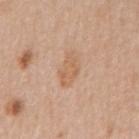follow-up — catalogued during a skin exam; not biopsied | illumination — white-light | acquisition — total-body-photography crop, ~15 mm field of view | size — about 4 mm | subject — male, aged approximately 70 | location — the chest.